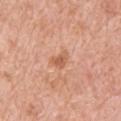The subject is a male about 55 years old. The recorded lesion diameter is about 2.5 mm. The lesion is located on the upper back. A lesion tile, about 15 mm wide, cut from a 3D total-body photograph.